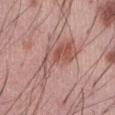{
  "lesion_size": {
    "long_diameter_mm_approx": 7.0
  },
  "lighting": "white-light",
  "patient": {
    "sex": "male",
    "age_approx": 55
  },
  "image": {
    "source": "total-body photography crop",
    "field_of_view_mm": 15
  },
  "site": "front of the torso"
}